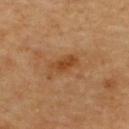biopsy_status: not biopsied; imaged during a skin examination
lesion_size:
  long_diameter_mm_approx: 3.5
lighting: cross-polarized
site: upper back
patient:
  age_approx: 60
automated_metrics:
  vs_skin_darker_L: 9.0
  vs_skin_contrast_norm: 8.0
  border_irregularity_0_10: 3.0
  color_variation_0_10: 2.5
image:
  source: total-body photography crop
  field_of_view_mm: 15